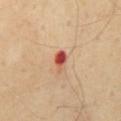Clinical impression: Imaged during a routine full-body skin examination; the lesion was not biopsied and no histopathology is available. Context: Imaged with cross-polarized lighting. Cropped from a whole-body photographic skin survey; the tile spans about 15 mm. An algorithmic analysis of the crop reported a mean CIELAB color near L≈55 a*≈33 b*≈33, a lesion–skin lightness drop of about 16, and a lesion-to-skin contrast of about 10 (normalized; higher = more distinct). It also reported a nevus-likeness score of about 0/100 and a lesion-detection confidence of about 100/100. Measured at roughly 2.5 mm in maximum diameter. From the mid back. A male patient in their mid- to late 50s.